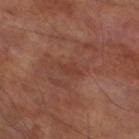• notes · catalogued during a skin exam; not biopsied
• body site · the left lower leg
• acquisition · 15 mm crop, total-body photography
• lesion size · ~3 mm (longest diameter)
• patient · male, aged 68–72
• automated lesion analysis · a lesion area of about 3.5 mm² and an outline eccentricity of about 0.8 (0 = round, 1 = elongated); an average lesion color of about L≈38 a*≈23 b*≈27 (CIELAB), about 5 CIELAB-L* units darker than the surrounding skin, and a lesion-to-skin contrast of about 4.5 (normalized; higher = more distinct)